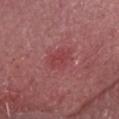The lesion was tiled from a total-body skin photograph and was not biopsied.
A 15 mm close-up tile from a total-body photography series done for melanoma screening.
From the head or neck.
The total-body-photography lesion software estimated a shape eccentricity near 0.75. The analysis additionally found an automated nevus-likeness rating near 10 out of 100 and a lesion-detection confidence of about 100/100.
Approximately 4 mm at its widest.
A male patient roughly 85 years of age or older.